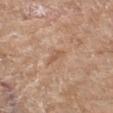{"biopsy_status": "not biopsied; imaged during a skin examination", "image": {"source": "total-body photography crop", "field_of_view_mm": 15}, "lesion_size": {"long_diameter_mm_approx": 2.5}, "lighting": "white-light", "site": "leg", "automated_metrics": {"eccentricity": 0.95, "shape_asymmetry": 0.3, "cielab_L": 56, "cielab_a": 20, "cielab_b": 32, "vs_skin_darker_L": 8.0, "vs_skin_contrast_norm": 5.5, "border_irregularity_0_10": 4.0, "color_variation_0_10": 0.0, "peripheral_color_asymmetry": 0.0, "nevus_likeness_0_100": 0}, "patient": {"sex": "female", "age_approx": 75}}